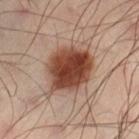This lesion was catalogued during total-body skin photography and was not selected for biopsy.
The lesion is located on the right lower leg.
The total-body-photography lesion software estimated a lesion color around L≈43 a*≈22 b*≈28 in CIELAB, a lesion–skin lightness drop of about 17, and a normalized border contrast of about 13.
A male patient, aged around 40.
The tile uses cross-polarized illumination.
A lesion tile, about 15 mm wide, cut from a 3D total-body photograph.
The lesion's longest dimension is about 7 mm.